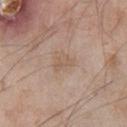{"biopsy_status": "not biopsied; imaged during a skin examination"}